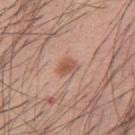Notes:
– workup · total-body-photography surveillance lesion; no biopsy
– automated lesion analysis · a lesion area of about 3.5 mm²; a border-irregularity rating of about 2.5/10, internal color variation of about 2.5 on a 0–10 scale, and peripheral color asymmetry of about 1; an automated nevus-likeness rating near 85 out of 100 and a lesion-detection confidence of about 100/100
– location · the mid back
– acquisition · total-body-photography crop, ~15 mm field of view
– lesion size · ≈2.5 mm
– patient · male, approximately 60 years of age